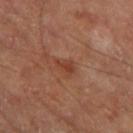| field | value |
|---|---|
| notes | total-body-photography surveillance lesion; no biopsy |
| illumination | cross-polarized |
| automated lesion analysis | peripheral color asymmetry of about 0.5 |
| image source | total-body-photography crop, ~15 mm field of view |
| patient | male, in their 70s |
| size | ≈2.5 mm |
| site | the left thigh |